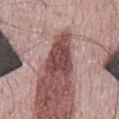Captured during whole-body skin photography for melanoma surveillance; the lesion was not biopsied.
A male patient, aged around 50.
Located on the mid back.
A close-up tile cropped from a whole-body skin photograph, about 15 mm across.
Measured at roughly 5 mm in maximum diameter.